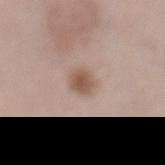<tbp_lesion>
  <biopsy_status>not biopsied; imaged during a skin examination</biopsy_status>
  <site>left lower leg</site>
  <patient>
    <sex>female</sex>
    <age_approx>65</age_approx>
  </patient>
  <image>
    <source>total-body photography crop</source>
    <field_of_view_mm>15</field_of_view_mm>
  </image>
  <lighting>white-light</lighting>
  <lesion_size>
    <long_diameter_mm_approx>2.5</long_diameter_mm_approx>
  </lesion_size>
  <automated_metrics>
    <eccentricity>0.6</eccentricity>
    <shape_asymmetry>0.25</shape_asymmetry>
    <cielab_L>54</cielab_L>
    <cielab_a>18</cielab_a>
    <cielab_b>26</cielab_b>
    <vs_skin_darker_L>11.0</vs_skin_darker_L>
    <vs_skin_contrast_norm>8.0</vs_skin_contrast_norm>
    <nevus_likeness_0_100>95</nevus_likeness_0_100>
    <lesion_detection_confidence_0_100>100</lesion_detection_confidence_0_100>
  </automated_metrics>
</tbp_lesion>